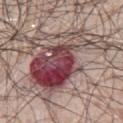Impression:
This lesion was catalogued during total-body skin photography and was not selected for biopsy.
Clinical summary:
A male subject, aged 68–72. On the chest. Imaged with white-light lighting. A lesion tile, about 15 mm wide, cut from a 3D total-body photograph. Automated image analysis of the tile measured a lesion color around L≈44 a*≈23 b*≈18 in CIELAB, roughly 18 lightness units darker than nearby skin, and a normalized lesion–skin contrast near 13. It also reported border irregularity of about 8 on a 0–10 scale, a within-lesion color-variation index near 10/10, and peripheral color asymmetry of about 5.